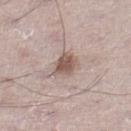{"biopsy_status": "not biopsied; imaged during a skin examination", "lighting": "white-light", "image": {"source": "total-body photography crop", "field_of_view_mm": 15}, "patient": {"sex": "male", "age_approx": 70}, "site": "leg", "automated_metrics": {"area_mm2_approx": 6.5, "eccentricity": 0.65, "shape_asymmetry": 0.15}}